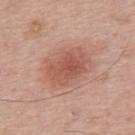A roughly 15 mm field-of-view crop from a total-body skin photograph. The tile uses white-light illumination. On the mid back. The total-body-photography lesion software estimated roughly 10 lightness units darker than nearby skin and a normalized lesion–skin contrast near 6.5. The software also gave a border-irregularity index near 2.5/10, internal color variation of about 3.5 on a 0–10 scale, and a peripheral color-asymmetry measure near 1. Longest diameter approximately 5 mm. A male patient, roughly 65 years of age.